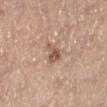Notes:
- biopsy status — total-body-photography surveillance lesion; no biopsy
- automated lesion analysis — a lesion–skin lightness drop of about 11 and a normalized lesion–skin contrast near 7.5; a border-irregularity index near 4.5/10, a within-lesion color-variation index near 3.5/10, and peripheral color asymmetry of about 1.5; an automated nevus-likeness rating near 65 out of 100 and a detector confidence of about 100 out of 100 that the crop contains a lesion
- image — 15 mm crop, total-body photography
- patient — female, in their mid-60s
- tile lighting — white-light
- location — the left lower leg